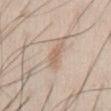Findings:
- workup · catalogued during a skin exam; not biopsied
- imaging modality · ~15 mm tile from a whole-body skin photo
- TBP lesion metrics · a border-irregularity index near 3.5/10, a color-variation rating of about 1.5/10, and a peripheral color-asymmetry measure near 0.5; a nevus-likeness score of about 20/100
- diameter · ≈3.5 mm
- tile lighting · white-light illumination
- subject · male, in their mid- to late 40s
- location · the chest